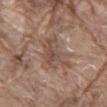Impression:
Part of a total-body skin-imaging series; this lesion was reviewed on a skin check and was not flagged for biopsy.
Image and clinical context:
Automated tile analysis of the lesion measured an area of roughly 10 mm², a shape eccentricity near 0.9, and two-axis asymmetry of about 0.35. The analysis additionally found border irregularity of about 5.5 on a 0–10 scale and peripheral color asymmetry of about 1.5. And it measured a nevus-likeness score of about 0/100 and lesion-presence confidence of about 60/100. A region of skin cropped from a whole-body photographic capture, roughly 15 mm wide. The lesion is located on the back. Approximately 5.5 mm at its widest. The patient is a male about 80 years old. The tile uses white-light illumination.